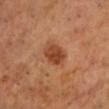biopsy status=total-body-photography surveillance lesion; no biopsy | tile lighting=cross-polarized | imaging modality=15 mm crop, total-body photography | site=the head or neck | patient=male, aged 63–67 | lesion size=≈4 mm.